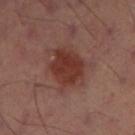The lesion was photographed on a routine skin check and not biopsied; there is no pathology result. On the left thigh. A 15 mm close-up tile from a total-body photography series done for melanoma screening.